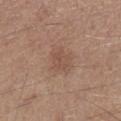workup = imaged on a skin check; not biopsied | lesion diameter = ≈2.5 mm | acquisition = ~15 mm crop, total-body skin-cancer survey | automated lesion analysis = a color-variation rating of about 1.5/10 and radial color variation of about 0.5 | location = the leg | patient = male, aged around 60.